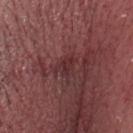Located on the head or neck. About 2.5 mm across. A male patient, aged 38 to 42. A roughly 15 mm field-of-view crop from a total-body skin photograph.